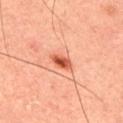Image and clinical context: About 3 mm across. The lesion is on the upper back. A male subject, about 45 years old. A lesion tile, about 15 mm wide, cut from a 3D total-body photograph. The lesion-visualizer software estimated a mean CIELAB color near L≈56 a*≈32 b*≈37, roughly 14 lightness units darker than nearby skin, and a normalized lesion–skin contrast near 9. The analysis additionally found border irregularity of about 2 on a 0–10 scale, a within-lesion color-variation index near 5.5/10, and a peripheral color-asymmetry measure near 1.5. The software also gave a nevus-likeness score of about 100/100 and a lesion-detection confidence of about 100/100.